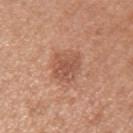Clinical impression:
Captured during whole-body skin photography for melanoma surveillance; the lesion was not biopsied.
Clinical summary:
The lesion is on the left upper arm. The patient is a female aged 48 to 52. A 15 mm close-up extracted from a 3D total-body photography capture.